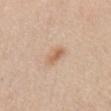| feature | finding |
|---|---|
| biopsy status | catalogued during a skin exam; not biopsied |
| body site | the front of the torso |
| image source | total-body-photography crop, ~15 mm field of view |
| patient | male, about 60 years old |
| size | ≈3.5 mm |